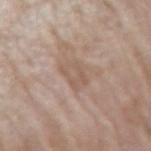A 15 mm close-up tile from a total-body photography series done for melanoma screening.
The lesion is located on the right forearm.
Automated tile analysis of the lesion measured a footprint of about 5.5 mm², a shape eccentricity near 0.8, and a symmetry-axis asymmetry near 0.25. And it measured roughly 7 lightness units darker than nearby skin and a lesion-to-skin contrast of about 5 (normalized; higher = more distinct). The analysis additionally found a border-irregularity rating of about 3/10.
The recorded lesion diameter is about 3 mm.
This is a white-light tile.
A female patient aged around 75.